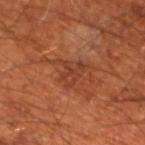Findings:
• biopsy status · no biopsy performed (imaged during a skin exam)
• acquisition · ~15 mm tile from a whole-body skin photo
• lighting · cross-polarized
• image-analysis metrics · a mean CIELAB color near L≈39 a*≈26 b*≈32, a lesion–skin lightness drop of about 7, and a normalized lesion–skin contrast near 6; border irregularity of about 5.5 on a 0–10 scale, a color-variation rating of about 2.5/10, and a peripheral color-asymmetry measure near 1; an automated nevus-likeness rating near 0 out of 100 and a detector confidence of about 100 out of 100 that the crop contains a lesion
• lesion diameter · ~3.5 mm (longest diameter)
• location · the leg
• patient · male, in their 70s